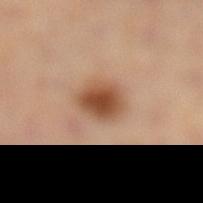Part of a total-body skin-imaging series; this lesion was reviewed on a skin check and was not flagged for biopsy. A 15 mm crop from a total-body photograph taken for skin-cancer surveillance. Captured under cross-polarized illumination. On the left leg. The subject is a male approximately 50 years of age.